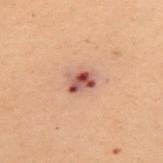workup = no biopsy performed (imaged during a skin exam)
lesion size = about 3 mm
acquisition = total-body-photography crop, ~15 mm field of view
automated lesion analysis = a lesion area of about 5.5 mm² and an eccentricity of roughly 0.65; a mean CIELAB color near L≈47 a*≈22 b*≈24, about 13 CIELAB-L* units darker than the surrounding skin, and a normalized lesion–skin contrast near 10; border irregularity of about 1.5 on a 0–10 scale, a color-variation rating of about 9.5/10, and peripheral color asymmetry of about 4
lighting = cross-polarized illumination
anatomic site = the upper back
patient = female, roughly 50 years of age